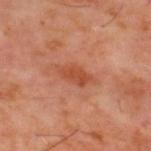{
  "biopsy_status": "not biopsied; imaged during a skin examination",
  "site": "upper back",
  "patient": {
    "sex": "male",
    "age_approx": 60
  },
  "lighting": "cross-polarized",
  "lesion_size": {
    "long_diameter_mm_approx": 3.5
  },
  "image": {
    "source": "total-body photography crop",
    "field_of_view_mm": 15
  }
}